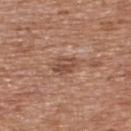Case summary:
- notes — imaged on a skin check; not biopsied
- tile lighting — white-light illumination
- subject — male, aged around 65
- anatomic site — the back
- image — ~15 mm crop, total-body skin-cancer survey
- size — about 2.5 mm